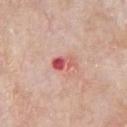  biopsy_status: not biopsied; imaged during a skin examination
  patient:
    sex: male
    age_approx: 65
  lesion_size:
    long_diameter_mm_approx: 3.0
  automated_metrics:
    area_mm2_approx: 4.0
    eccentricity: 0.85
    shape_asymmetry: 0.35
    cielab_L: 57
    cielab_a: 34
    cielab_b: 26
    vs_skin_darker_L: 13.0
  image:
    source: total-body photography crop
    field_of_view_mm: 15
  lighting: white-light
  site: chest